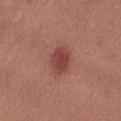<case>
  <biopsy_status>not biopsied; imaged during a skin examination</biopsy_status>
  <image>
    <source>total-body photography crop</source>
    <field_of_view_mm>15</field_of_view_mm>
  </image>
  <patient>
    <sex>female</sex>
    <age_approx>55</age_approx>
  </patient>
  <site>leg</site>
</case>